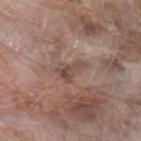Impression: The lesion was tiled from a total-body skin photograph and was not biopsied. Image and clinical context: From the left forearm. This is a white-light tile. A female patient in their mid- to late 70s. This image is a 15 mm lesion crop taken from a total-body photograph.